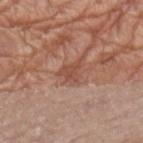lesion_size:
  long_diameter_mm_approx: 3.5
lighting: white-light
site: left upper arm
patient:
  sex: female
  age_approx: 75
image:
  source: total-body photography crop
  field_of_view_mm: 15
automated_metrics:
  area_mm2_approx: 4.5
  eccentricity: 0.7
  shape_asymmetry: 0.5
  border_irregularity_0_10: 5.0
  color_variation_0_10: 2.0
  peripheral_color_asymmetry: 1.0